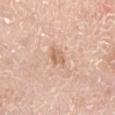Assessment: Part of a total-body skin-imaging series; this lesion was reviewed on a skin check and was not flagged for biopsy. Acquisition and patient details: From the left lower leg. Measured at roughly 2.5 mm in maximum diameter. A male subject, aged around 60. Cropped from a total-body skin-imaging series; the visible field is about 15 mm. Captured under white-light illumination.